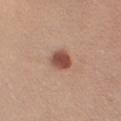The subject is a female aged 43 to 47.
Captured under white-light illumination.
From the right upper arm.
The total-body-photography lesion software estimated a footprint of about 5.5 mm² and an eccentricity of roughly 0.65. And it measured border irregularity of about 1.5 on a 0–10 scale and radial color variation of about 1. It also reported an automated nevus-likeness rating near 95 out of 100.
Cropped from a whole-body photographic skin survey; the tile spans about 15 mm.
The lesion's longest dimension is about 3 mm.